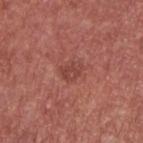| field | value |
|---|---|
| biopsy status | total-body-photography surveillance lesion; no biopsy |
| anatomic site | the upper back |
| imaging modality | ~15 mm tile from a whole-body skin photo |
| patient | male, in their mid- to late 60s |
| size | ~3 mm (longest diameter) |
| tile lighting | white-light illumination |